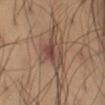follow-up: imaged on a skin check; not biopsied | location: the left thigh | patient: male, about 40 years old | illumination: cross-polarized illumination | acquisition: 15 mm crop, total-body photography.